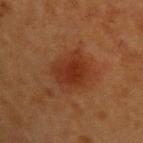workup: no biopsy performed (imaged during a skin exam).